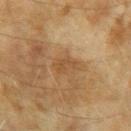Imaged during a routine full-body skin examination; the lesion was not biopsied and no histopathology is available. From the right upper arm. Captured under cross-polarized illumination. Measured at roughly 3.5 mm in maximum diameter. The subject is a female about 60 years old. Cropped from a total-body skin-imaging series; the visible field is about 15 mm.